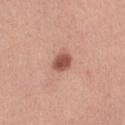No biopsy was performed on this lesion — it was imaged during a full skin examination and was not determined to be concerning. Cropped from a total-body skin-imaging series; the visible field is about 15 mm. Approximately 2.5 mm at its widest. Automated tile analysis of the lesion measured border irregularity of about 1.5 on a 0–10 scale and peripheral color asymmetry of about 1. The software also gave a classifier nevus-likeness of about 100/100 and a detector confidence of about 100 out of 100 that the crop contains a lesion. A female patient, about 30 years old. The lesion is on the right thigh.